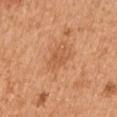Assessment:
This lesion was catalogued during total-body skin photography and was not selected for biopsy.